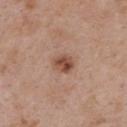Image and clinical context: The subject is a female approximately 30 years of age. Imaged with white-light lighting. A 15 mm crop from a total-body photograph taken for skin-cancer surveillance. Located on the back.